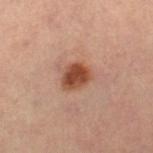Impression: Captured during whole-body skin photography for melanoma surveillance; the lesion was not biopsied. Image and clinical context: This is a cross-polarized tile. A lesion tile, about 15 mm wide, cut from a 3D total-body photograph. A male subject approximately 55 years of age. The lesion's longest dimension is about 3 mm. Located on the right thigh.